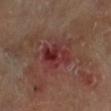| key | value |
|---|---|
| lesion diameter | ≈4 mm |
| subject | male, approximately 70 years of age |
| site | the left lower leg |
| image | ~15 mm tile from a whole-body skin photo |
| tile lighting | cross-polarized |